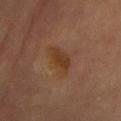<tbp_lesion>
<site>front of the torso</site>
<lesion_size>
  <long_diameter_mm_approx>4.5</long_diameter_mm_approx>
</lesion_size>
<patient>
  <sex>female</sex>
  <age_approx>60</age_approx>
</patient>
<automated_metrics>
  <cielab_L>33</cielab_L>
  <cielab_a>17</cielab_a>
  <cielab_b>28</cielab_b>
  <border_irregularity_0_10>3.0</border_irregularity_0_10>
  <color_variation_0_10>3.5</color_variation_0_10>
  <peripheral_color_asymmetry>1.0</peripheral_color_asymmetry>
</automated_metrics>
<lighting>cross-polarized</lighting>
<image>
  <source>total-body photography crop</source>
  <field_of_view_mm>15</field_of_view_mm>
</image>
</tbp_lesion>